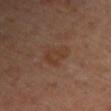No biopsy was performed on this lesion — it was imaged during a full skin examination and was not determined to be concerning. A roughly 15 mm field-of-view crop from a total-body skin photograph. Located on the left upper arm. A male patient, in their mid-60s. The tile uses cross-polarized illumination. Approximately 4 mm at its widest.